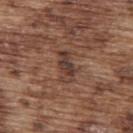The lesion was tiled from a total-body skin photograph and was not biopsied. A male subject aged 73–77. The lesion is located on the upper back. A 15 mm close-up extracted from a 3D total-body photography capture.